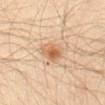This lesion was catalogued during total-body skin photography and was not selected for biopsy. Imaged with cross-polarized lighting. Located on the abdomen. A male subject, aged 38 to 42. A 15 mm close-up extracted from a 3D total-body photography capture. Measured at roughly 3 mm in maximum diameter. The lesion-visualizer software estimated a footprint of about 6 mm², an outline eccentricity of about 0.75 (0 = round, 1 = elongated), and a shape-asymmetry score of about 0.2 (0 = symmetric). It also reported an average lesion color of about L≈63 a*≈22 b*≈37 (CIELAB), a lesion–skin lightness drop of about 12, and a normalized border contrast of about 8. And it measured border irregularity of about 1.5 on a 0–10 scale, a color-variation rating of about 5.5/10, and a peripheral color-asymmetry measure near 2.